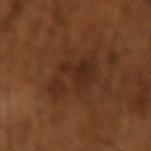biopsy status = imaged on a skin check; not biopsied | image = 15 mm crop, total-body photography | illumination = cross-polarized illumination | patient = male, aged 63–67 | body site = the arm.